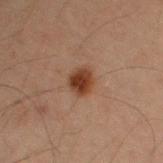notes: catalogued during a skin exam; not biopsied | anatomic site: the right upper arm | subject: male, aged around 60 | image: total-body-photography crop, ~15 mm field of view.